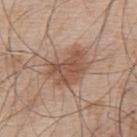workup — imaged on a skin check; not biopsied
subject — male, aged 53 to 57
image — 15 mm crop, total-body photography
lesion size — ≈5 mm
anatomic site — the back